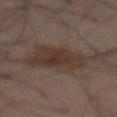| key | value |
|---|---|
| site | the lower back |
| patient | male, roughly 65 years of age |
| lesion size | ~7.5 mm (longest diameter) |
| imaging modality | ~15 mm crop, total-body skin-cancer survey |
| illumination | cross-polarized |